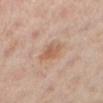Measured at roughly 3 mm in maximum diameter. A region of skin cropped from a whole-body photographic capture, roughly 15 mm wide. From the right leg. The patient is a female approximately 40 years of age. The lesion-visualizer software estimated an outline eccentricity of about 0.55 (0 = round, 1 = elongated). The software also gave border irregularity of about 2 on a 0–10 scale, internal color variation of about 2.5 on a 0–10 scale, and peripheral color asymmetry of about 1.